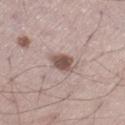Notes:
* biopsy status: catalogued during a skin exam; not biopsied
* subject: male, aged around 45
* body site: the left thigh
* acquisition: total-body-photography crop, ~15 mm field of view